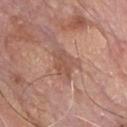Assessment: Imaged during a routine full-body skin examination; the lesion was not biopsied and no histopathology is available. Clinical summary: The patient is a male about 60 years old. Measured at roughly 4 mm in maximum diameter. This image is a 15 mm lesion crop taken from a total-body photograph. An algorithmic analysis of the crop reported a border-irregularity rating of about 3.5/10, internal color variation of about 2.5 on a 0–10 scale, and a peripheral color-asymmetry measure near 0.5. The tile uses white-light illumination. Located on the chest.